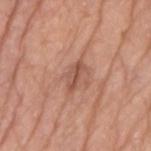workup = imaged on a skin check; not biopsied | lighting = white-light | body site = the right upper arm | acquisition = 15 mm crop, total-body photography | patient = male, aged 78 to 82.